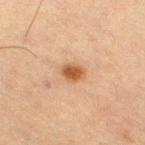patient = male, aged 63 to 67 | illumination = cross-polarized | anatomic site = the right thigh | size = ≈3 mm | image source = total-body-photography crop, ~15 mm field of view.